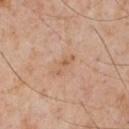{"biopsy_status": "not biopsied; imaged during a skin examination", "lesion_size": {"long_diameter_mm_approx": 3.0}, "lighting": "white-light", "patient": {"sex": "male", "age_approx": 60}, "automated_metrics": {"area_mm2_approx": 3.0, "shape_asymmetry": 0.35, "cielab_L": 60, "cielab_a": 21, "cielab_b": 34, "vs_skin_darker_L": 7.0, "vs_skin_contrast_norm": 5.5, "nevus_likeness_0_100": 0, "lesion_detection_confidence_0_100": 100}, "site": "chest", "image": {"source": "total-body photography crop", "field_of_view_mm": 15}}